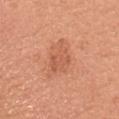Assessment:
Recorded during total-body skin imaging; not selected for excision or biopsy.
Clinical summary:
Imaged with white-light lighting. A female patient, roughly 45 years of age. An algorithmic analysis of the crop reported a shape eccentricity near 0.85 and a symmetry-axis asymmetry near 0.4. The analysis additionally found a lesion color around L≈58 a*≈28 b*≈35 in CIELAB and a lesion–skin lightness drop of about 8. The analysis additionally found a border-irregularity rating of about 5/10 and a color-variation rating of about 2/10. A 15 mm close-up extracted from a 3D total-body photography capture. On the head or neck.